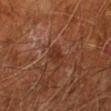Q: Was a biopsy performed?
A: catalogued during a skin exam; not biopsied
Q: What are the patient's age and sex?
A: male, aged around 65
Q: What kind of image is this?
A: total-body-photography crop, ~15 mm field of view
Q: Where on the body is the lesion?
A: the leg
Q: Automated lesion metrics?
A: a lesion area of about 4 mm² and an eccentricity of roughly 0.8; a mean CIELAB color near L≈31 a*≈23 b*≈28, roughly 7 lightness units darker than nearby skin, and a normalized lesion–skin contrast near 6.5; border irregularity of about 2.5 on a 0–10 scale, a color-variation rating of about 1.5/10, and radial color variation of about 0.5; a detector confidence of about 100 out of 100 that the crop contains a lesion
Q: How large is the lesion?
A: ≈3 mm